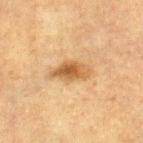Imaged during a routine full-body skin examination; the lesion was not biopsied and no histopathology is available.
The lesion is on the right lower leg.
This is a cross-polarized tile.
A roughly 15 mm field-of-view crop from a total-body skin photograph.
About 4.5 mm across.
The subject is a female in their mid-50s.